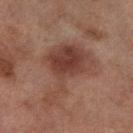notes = no biopsy performed (imaged during a skin exam)
subject = female, aged approximately 60
tile lighting = cross-polarized
automated metrics = an average lesion color of about L≈35 a*≈19 b*≈22 (CIELAB), roughly 8 lightness units darker than nearby skin, and a normalized lesion–skin contrast near 7.5; a border-irregularity index near 7/10, a within-lesion color-variation index near 4.5/10, and radial color variation of about 1.5; a nevus-likeness score of about 5/100 and lesion-presence confidence of about 100/100
anatomic site = the left thigh
imaging modality = total-body-photography crop, ~15 mm field of view